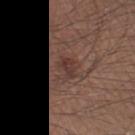  biopsy_status: not biopsied; imaged during a skin examination
  lighting: white-light
  site: left thigh
  lesion_size:
    long_diameter_mm_approx: 2.5
  patient:
    sex: male
    age_approx: 45
  image:
    source: total-body photography crop
    field_of_view_mm: 15
  automated_metrics:
    area_mm2_approx: 4.0
    eccentricity: 0.7
    shape_asymmetry: 0.35